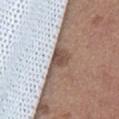patient: female, aged 63 to 67 | tile lighting: white-light | imaging modality: ~15 mm tile from a whole-body skin photo | body site: the abdomen | diameter: ~4 mm (longest diameter) | automated lesion analysis: an area of roughly 6.5 mm², a shape eccentricity near 0.8, and two-axis asymmetry of about 0.45; a nevus-likeness score of about 0/100 and a detector confidence of about 100 out of 100 that the crop contains a lesion.